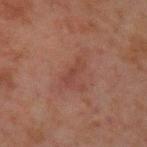Recorded during total-body skin imaging; not selected for excision or biopsy.
This image is a 15 mm lesion crop taken from a total-body photograph.
The subject is a male aged around 30.
From the left upper arm.